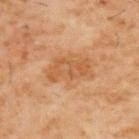Captured during whole-body skin photography for melanoma surveillance; the lesion was not biopsied. The lesion is on the upper back. The subject is a male approximately 60 years of age. The total-body-photography lesion software estimated a lesion area of about 9 mm², an outline eccentricity of about 0.9 (0 = round, 1 = elongated), and two-axis asymmetry of about 0.3. And it measured roughly 8 lightness units darker than nearby skin and a normalized border contrast of about 6. It also reported a border-irregularity index near 4/10, a within-lesion color-variation index near 3/10, and radial color variation of about 1. Captured under cross-polarized illumination. A lesion tile, about 15 mm wide, cut from a 3D total-body photograph. Measured at roughly 5 mm in maximum diameter.